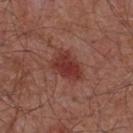From the chest.
Captured under white-light illumination.
A male subject, approximately 55 years of age.
Approximately 4.5 mm at its widest.
A 15 mm close-up tile from a total-body photography series done for melanoma screening.